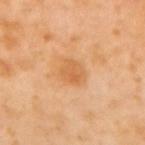* follow-up · imaged on a skin check; not biopsied
* subject · female, aged 53 to 57
* imaging modality · ~15 mm crop, total-body skin-cancer survey
* body site · the upper back